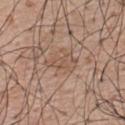Q: Was a biopsy performed?
A: catalogued during a skin exam; not biopsied
Q: Patient demographics?
A: male, about 65 years old
Q: How large is the lesion?
A: ≈4 mm
Q: What is the imaging modality?
A: total-body-photography crop, ~15 mm field of view
Q: What lighting was used for the tile?
A: white-light illumination
Q: What is the anatomic site?
A: the upper back